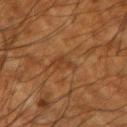{"biopsy_status": "not biopsied; imaged during a skin examination", "image": {"source": "total-body photography crop", "field_of_view_mm": 15}, "patient": {"sex": "male", "age_approx": 65}, "lesion_size": {"long_diameter_mm_approx": 3.0}, "site": "left upper arm", "automated_metrics": {"cielab_L": 31, "cielab_a": 18, "cielab_b": 29, "vs_skin_darker_L": 5.0, "vs_skin_contrast_norm": 5.0, "border_irregularity_0_10": 3.5, "peripheral_color_asymmetry": 0.5}, "lighting": "cross-polarized"}